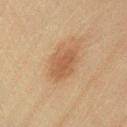follow-up — no biopsy performed (imaged during a skin exam); acquisition — ~15 mm crop, total-body skin-cancer survey; anatomic site — the left lower leg; size — ~4.5 mm (longest diameter); subject — female, aged around 50; illumination — cross-polarized illumination.